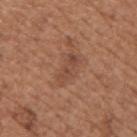| feature | finding |
|---|---|
| body site | the upper back |
| lighting | white-light |
| patient | male, in their mid-60s |
| image source | total-body-photography crop, ~15 mm field of view |
| automated lesion analysis | a lesion area of about 4.5 mm², an eccentricity of roughly 0.9, and two-axis asymmetry of about 0.35; border irregularity of about 4.5 on a 0–10 scale, a color-variation rating of about 2/10, and radial color variation of about 0.5; a lesion-detection confidence of about 100/100 |